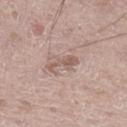Part of a total-body skin-imaging series; this lesion was reviewed on a skin check and was not flagged for biopsy.
On the leg.
This is a white-light tile.
Cropped from a total-body skin-imaging series; the visible field is about 15 mm.
Measured at roughly 4 mm in maximum diameter.
A male subject, aged 63–67.
Automated image analysis of the tile measured a lesion area of about 4.5 mm², an eccentricity of roughly 0.95, and a shape-asymmetry score of about 0.5 (0 = symmetric). And it measured a mean CIELAB color near L≈57 a*≈17 b*≈24, a lesion–skin lightness drop of about 9, and a normalized lesion–skin contrast near 6.5. The software also gave a nevus-likeness score of about 0/100 and a detector confidence of about 100 out of 100 that the crop contains a lesion.